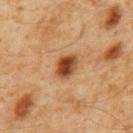biopsy_status: not biopsied; imaged during a skin examination
site: mid back
lighting: cross-polarized
patient:
  sex: male
  age_approx: 60
automated_metrics:
  border_irregularity_0_10: 2.0
  color_variation_0_10: 6.5
  nevus_likeness_0_100: 100
  lesion_detection_confidence_0_100: 100
image:
  source: total-body photography crop
  field_of_view_mm: 15
lesion_size:
  long_diameter_mm_approx: 3.0